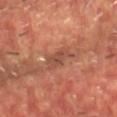Assessment:
Recorded during total-body skin imaging; not selected for excision or biopsy.
Image and clinical context:
Automated image analysis of the tile measured a lesion area of about 6 mm², an outline eccentricity of about 0.85 (0 = round, 1 = elongated), and a shape-asymmetry score of about 0.25 (0 = symmetric). The software also gave a mean CIELAB color near L≈44 a*≈23 b*≈28, roughly 7 lightness units darker than nearby skin, and a normalized border contrast of about 6. The analysis additionally found a classifier nevus-likeness of about 0/100. A close-up tile cropped from a whole-body skin photograph, about 15 mm across. About 3.5 mm across. The lesion is located on the back. A male patient, roughly 65 years of age.